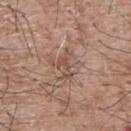biopsy_status: not biopsied; imaged during a skin examination
patient:
  sex: male
  age_approx: 65
image:
  source: total-body photography crop
  field_of_view_mm: 15
site: upper back